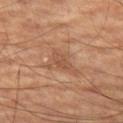{"biopsy_status": "not biopsied; imaged during a skin examination"}